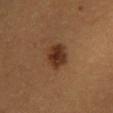Q: Is there a histopathology result?
A: total-body-photography surveillance lesion; no biopsy
Q: What is the lesion's diameter?
A: ~3.5 mm (longest diameter)
Q: How was this image acquired?
A: 15 mm crop, total-body photography
Q: How was the tile lit?
A: cross-polarized
Q: Automated lesion metrics?
A: a lesion area of about 8.5 mm² and two-axis asymmetry of about 0.15; an average lesion color of about L≈32 a*≈19 b*≈29 (CIELAB), about 11 CIELAB-L* units darker than the surrounding skin, and a normalized lesion–skin contrast near 10; a border-irregularity index near 1.5/10 and radial color variation of about 1; an automated nevus-likeness rating near 95 out of 100 and a lesion-detection confidence of about 100/100
Q: Patient demographics?
A: male, aged 18–22
Q: Lesion location?
A: the chest